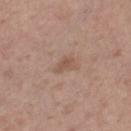Part of a total-body skin-imaging series; this lesion was reviewed on a skin check and was not flagged for biopsy. On the right thigh. This image is a 15 mm lesion crop taken from a total-body photograph. The patient is a female roughly 75 years of age. About 3 mm across. An algorithmic analysis of the crop reported an eccentricity of roughly 0.75 and a symmetry-axis asymmetry near 0.4. And it measured a color-variation rating of about 1.5/10 and a peripheral color-asymmetry measure near 0.5. It also reported a nevus-likeness score of about 0/100.